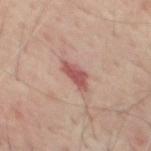  biopsy_status: not biopsied; imaged during a skin examination
  site: chest
  image:
    source: total-body photography crop
    field_of_view_mm: 15
  patient:
    sex: male
    age_approx: 65